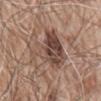Q: Was a biopsy performed?
A: imaged on a skin check; not biopsied
Q: What is the imaging modality?
A: 15 mm crop, total-body photography
Q: Illumination type?
A: white-light illumination
Q: Who is the patient?
A: male, aged around 80
Q: Lesion location?
A: the mid back
Q: How large is the lesion?
A: about 8 mm